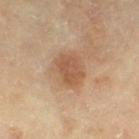The lesion was tiled from a total-body skin photograph and was not biopsied. The lesion's longest dimension is about 4 mm. A roughly 15 mm field-of-view crop from a total-body skin photograph. The patient is a male aged 63–67. From the right thigh.